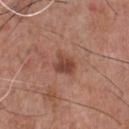Context:
This image is a 15 mm lesion crop taken from a total-body photograph. On the chest. A male patient, aged 68 to 72. Automated tile analysis of the lesion measured a lesion color around L≈44 a*≈24 b*≈27 in CIELAB, a lesion–skin lightness drop of about 11, and a normalized border contrast of about 8.5. It also reported a classifier nevus-likeness of about 70/100.